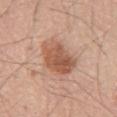Imaged during a routine full-body skin examination; the lesion was not biopsied and no histopathology is available. The lesion is located on the abdomen. A male subject, approximately 60 years of age. A close-up tile cropped from a whole-body skin photograph, about 15 mm across.